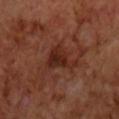Impression:
The lesion was tiled from a total-body skin photograph and was not biopsied.
Acquisition and patient details:
This is a cross-polarized tile. About 4.5 mm across. On the chest. Automated tile analysis of the lesion measured a lesion area of about 8 mm² and an eccentricity of roughly 0.65. The software also gave roughly 8 lightness units darker than nearby skin and a normalized border contrast of about 8.5. A lesion tile, about 15 mm wide, cut from a 3D total-body photograph. The subject is a male approximately 70 years of age.